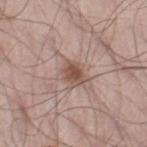  biopsy_status: not biopsied; imaged during a skin examination
  image:
    source: total-body photography crop
    field_of_view_mm: 15
  patient:
    sex: male
    age_approx: 55
  lighting: white-light
  automated_metrics:
    area_mm2_approx: 4.5
    shape_asymmetry: 0.25
    cielab_L: 51
    cielab_a: 19
    cielab_b: 26
    vs_skin_darker_L: 11.0
    vs_skin_contrast_norm: 8.5
    lesion_detection_confidence_0_100: 100
  site: leg
  lesion_size:
    long_diameter_mm_approx: 2.5